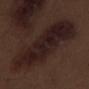Recorded during total-body skin imaging; not selected for excision or biopsy. A close-up tile cropped from a whole-body skin photograph, about 15 mm across. The lesion is on the right thigh. Captured under white-light illumination. A male patient about 70 years old. The recorded lesion diameter is about 11 mm. The lesion-visualizer software estimated a within-lesion color-variation index near 5/10 and peripheral color asymmetry of about 1.5.